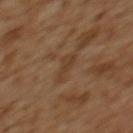Imaged with cross-polarized lighting.
The lesion-visualizer software estimated an area of roughly 4 mm², an eccentricity of roughly 0.85, and two-axis asymmetry of about 0.4.
A region of skin cropped from a whole-body photographic capture, roughly 15 mm wide.
Approximately 3 mm at its widest.
The lesion is located on the upper back.
The subject is a female aged around 55.